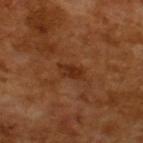Part of a total-body skin-imaging series; this lesion was reviewed on a skin check and was not flagged for biopsy. The recorded lesion diameter is about 3 mm. A male patient roughly 65 years of age. This image is a 15 mm lesion crop taken from a total-body photograph.